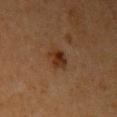• notes: imaged on a skin check; not biopsied
• anatomic site: the left upper arm
• subject: female, aged 38 to 42
• image source: ~15 mm tile from a whole-body skin photo
• tile lighting: cross-polarized illumination
• diameter: about 3 mm